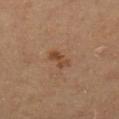Imaged during a routine full-body skin examination; the lesion was not biopsied and no histopathology is available.
A female patient roughly 60 years of age.
Measured at roughly 3 mm in maximum diameter.
The tile uses cross-polarized illumination.
An algorithmic analysis of the crop reported a lesion area of about 4 mm², a shape eccentricity near 0.9, and two-axis asymmetry of about 0.25. And it measured a border-irregularity rating of about 3/10, a color-variation rating of about 2/10, and radial color variation of about 0.5. The software also gave a detector confidence of about 100 out of 100 that the crop contains a lesion.
From the left lower leg.
A 15 mm close-up tile from a total-body photography series done for melanoma screening.